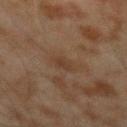The lesion was photographed on a routine skin check and not biopsied; there is no pathology result.
From the arm.
A male patient in their mid- to late 40s.
Measured at roughly 2.5 mm in maximum diameter.
A 15 mm close-up tile from a total-body photography series done for melanoma screening.
Imaged with cross-polarized lighting.